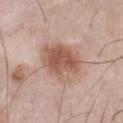A male patient in their mid- to late 50s. The tile uses white-light illumination. The lesion is located on the chest. Longest diameter approximately 5.5 mm. Cropped from a total-body skin-imaging series; the visible field is about 15 mm.